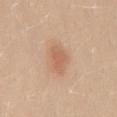workup — no biopsy performed (imaged during a skin exam) | anatomic site — the lower back | patient — female, aged 18–22 | image — 15 mm crop, total-body photography.